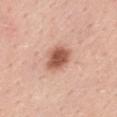Clinical impression:
Part of a total-body skin-imaging series; this lesion was reviewed on a skin check and was not flagged for biopsy.
Background:
This is a white-light tile. A roughly 15 mm field-of-view crop from a total-body skin photograph. Automated image analysis of the tile measured a footprint of about 8 mm², a shape eccentricity near 0.5, and a symmetry-axis asymmetry near 0.25. The analysis additionally found a lesion–skin lightness drop of about 15. The analysis additionally found an automated nevus-likeness rating near 100 out of 100 and lesion-presence confidence of about 100/100. A male patient, aged around 55. Located on the back.